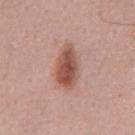notes: imaged on a skin check; not biopsied | image: ~15 mm crop, total-body skin-cancer survey | patient: male, approximately 55 years of age | automated metrics: a shape eccentricity near 0.85 and a symmetry-axis asymmetry near 0.15; a border-irregularity rating of about 2/10, a color-variation rating of about 5.5/10, and a peripheral color-asymmetry measure near 1.5; a classifier nevus-likeness of about 95/100.